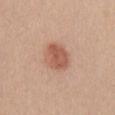Findings:
* follow-up · no biopsy performed (imaged during a skin exam)
* patient · female, aged 38 to 42
* anatomic site · the mid back
* image source · ~15 mm crop, total-body skin-cancer survey